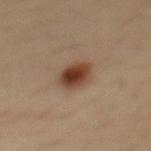  lesion_size:
    long_diameter_mm_approx: 4.0
  image:
    source: total-body photography crop
    field_of_view_mm: 15
  patient:
    sex: female
    age_approx: 35
  site: mid back
  automated_metrics:
    border_irregularity_0_10: 1.5
    color_variation_0_10: 6.0
    peripheral_color_asymmetry: 1.5